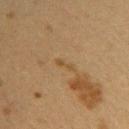Impression: Part of a total-body skin-imaging series; this lesion was reviewed on a skin check and was not flagged for biopsy. Clinical summary: Imaged with cross-polarized lighting. A female subject in their 40s. An algorithmic analysis of the crop reported an automated nevus-likeness rating near 0 out of 100 and lesion-presence confidence of about 95/100. Located on the right upper arm. A region of skin cropped from a whole-body photographic capture, roughly 15 mm wide.